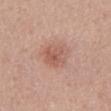The lesion was photographed on a routine skin check and not biopsied; there is no pathology result.
The lesion is on the abdomen.
Automated tile analysis of the lesion measured an area of roughly 12 mm², an outline eccentricity of about 0.7 (0 = round, 1 = elongated), and two-axis asymmetry of about 0.2. The analysis additionally found peripheral color asymmetry of about 1.5. The analysis additionally found a nevus-likeness score of about 50/100.
Cropped from a whole-body photographic skin survey; the tile spans about 15 mm.
The subject is a male aged around 50.
The tile uses white-light illumination.